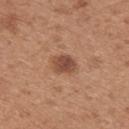Case summary:
• notes — catalogued during a skin exam; not biopsied